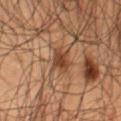notes: imaged on a skin check; not biopsied
patient: male, aged approximately 50
location: the lower back
imaging modality: 15 mm crop, total-body photography